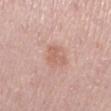| field | value |
|---|---|
| biopsy status | total-body-photography surveillance lesion; no biopsy |
| site | the leg |
| imaging modality | total-body-photography crop, ~15 mm field of view |
| diameter | about 2.5 mm |
| illumination | white-light |
| subject | female, about 40 years old |